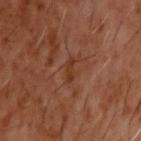biopsy status — imaged on a skin check; not biopsied
diameter — ≈2.5 mm
body site — the head or neck
image — total-body-photography crop, ~15 mm field of view
subject — male, aged 58–62